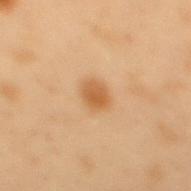No biopsy was performed on this lesion — it was imaged during a full skin examination and was not determined to be concerning.
Imaged with cross-polarized lighting.
Cropped from a total-body skin-imaging series; the visible field is about 15 mm.
Measured at roughly 3 mm in maximum diameter.
Automated tile analysis of the lesion measured a lesion color around L≈48 a*≈18 b*≈35 in CIELAB, about 8 CIELAB-L* units darker than the surrounding skin, and a normalized border contrast of about 7.5. And it measured a within-lesion color-variation index near 2/10 and peripheral color asymmetry of about 0.5. The software also gave a classifier nevus-likeness of about 95/100.
A male patient, aged 53–57.
The lesion is on the mid back.